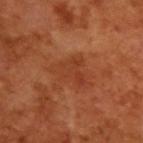Recorded during total-body skin imaging; not selected for excision or biopsy.
A male subject in their mid-60s.
A 15 mm crop from a total-body photograph taken for skin-cancer surveillance.